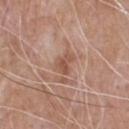Background: On the chest. A male patient in their mid- to late 60s. A 15 mm close-up extracted from a 3D total-body photography capture.